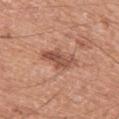Clinical impression:
Recorded during total-body skin imaging; not selected for excision or biopsy.
Background:
Imaged with white-light lighting. Located on the left thigh. The lesion's longest dimension is about 4 mm. A region of skin cropped from a whole-body photographic capture, roughly 15 mm wide. The total-body-photography lesion software estimated a border-irregularity rating of about 4.5/10, a color-variation rating of about 3.5/10, and a peripheral color-asymmetry measure near 1.5. It also reported a classifier nevus-likeness of about 15/100. A male patient, roughly 55 years of age.